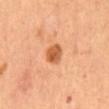workup: imaged on a skin check; not biopsied
location: the abdomen
imaging modality: 15 mm crop, total-body photography
illumination: cross-polarized
patient: female